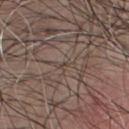Q: Is there a histopathology result?
A: total-body-photography surveillance lesion; no biopsy
Q: Lesion size?
A: about 1.5 mm
Q: Where on the body is the lesion?
A: the front of the torso
Q: How was this image acquired?
A: total-body-photography crop, ~15 mm field of view
Q: What are the patient's age and sex?
A: male, aged around 45